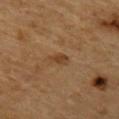follow-up: total-body-photography surveillance lesion; no biopsy
image source: ~15 mm crop, total-body skin-cancer survey
TBP lesion metrics: an average lesion color of about L≈35 a*≈16 b*≈29 (CIELAB), a lesion–skin lightness drop of about 7, and a lesion-to-skin contrast of about 6.5 (normalized; higher = more distinct); a classifier nevus-likeness of about 70/100 and a lesion-detection confidence of about 100/100
subject: male, aged around 85 or older
anatomic site: the mid back
lighting: cross-polarized illumination
lesion diameter: ≈2.5 mm